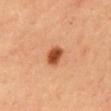<tbp_lesion>
  <biopsy_status>not biopsied; imaged during a skin examination</biopsy_status>
  <lesion_size>
    <long_diameter_mm_approx>2.5</long_diameter_mm_approx>
  </lesion_size>
  <site>mid back</site>
  <lighting>cross-polarized</lighting>
  <patient>
    <sex>male</sex>
    <age_approx>35</age_approx>
  </patient>
  <image>
    <source>total-body photography crop</source>
    <field_of_view_mm>15</field_of_view_mm>
  </image>
  <automated_metrics>
    <eccentricity>0.55</eccentricity>
    <shape_asymmetry>0.2</shape_asymmetry>
    <nevus_likeness_0_100>100</nevus_likeness_0_100>
  </automated_metrics>
</tbp_lesion>